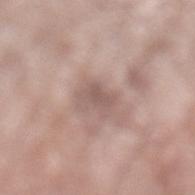{
  "biopsy_status": "not biopsied; imaged during a skin examination",
  "image": {
    "source": "total-body photography crop",
    "field_of_view_mm": 15
  },
  "site": "left lower leg",
  "lighting": "white-light",
  "patient": {
    "sex": "female",
    "age_approx": 75
  }
}